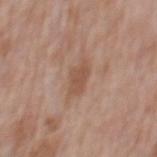Recorded during total-body skin imaging; not selected for excision or biopsy.
Imaged with white-light lighting.
A 15 mm close-up tile from a total-body photography series done for melanoma screening.
Automated tile analysis of the lesion measured an average lesion color of about L≈52 a*≈20 b*≈29 (CIELAB) and a lesion–skin lightness drop of about 8.
A male subject, aged 68–72.
On the mid back.